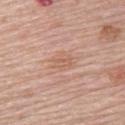Impression:
Part of a total-body skin-imaging series; this lesion was reviewed on a skin check and was not flagged for biopsy.
Image and clinical context:
From the upper back. A female subject aged 63 to 67. A roughly 15 mm field-of-view crop from a total-body skin photograph.